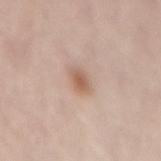Findings:
* notes: catalogued during a skin exam; not biopsied
* diameter: ~2.5 mm (longest diameter)
* image source: ~15 mm tile from a whole-body skin photo
* image-analysis metrics: border irregularity of about 2 on a 0–10 scale
* site: the mid back
* subject: female, aged around 65
* illumination: white-light illumination